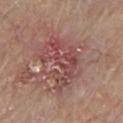Clinical impression:
Recorded during total-body skin imaging; not selected for excision or biopsy.
Background:
A male subject in their 80s. Cropped from a total-body skin-imaging series; the visible field is about 15 mm. The lesion-visualizer software estimated an eccentricity of roughly 0.75 and a shape-asymmetry score of about 0.45 (0 = symmetric). And it measured an average lesion color of about L≈47 a*≈24 b*≈22 (CIELAB) and a lesion–skin lightness drop of about 9. The software also gave a classifier nevus-likeness of about 0/100 and lesion-presence confidence of about 100/100. Measured at roughly 6.5 mm in maximum diameter. The lesion is on the chest. This is a white-light tile.